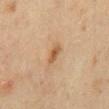Recorded during total-body skin imaging; not selected for excision or biopsy.